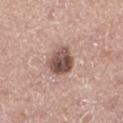Recorded during total-body skin imaging; not selected for excision or biopsy.
The patient is a male about 65 years old.
From the left lower leg.
The tile uses white-light illumination.
A 15 mm crop from a total-body photograph taken for skin-cancer surveillance.